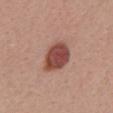| key | value |
|---|---|
| notes | total-body-photography surveillance lesion; no biopsy |
| body site | the back |
| subject | female, roughly 30 years of age |
| diameter | ≈4.5 mm |
| lighting | white-light |
| acquisition | ~15 mm crop, total-body skin-cancer survey |